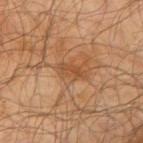Impression:
Imaged during a routine full-body skin examination; the lesion was not biopsied and no histopathology is available.
Acquisition and patient details:
This image is a 15 mm lesion crop taken from a total-body photograph. A male subject, roughly 65 years of age. On the arm.